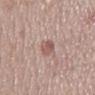notes: catalogued during a skin exam; not biopsied
imaging modality: ~15 mm tile from a whole-body skin photo
lesion diameter: about 3.5 mm
anatomic site: the mid back
subject: male, in their 70s